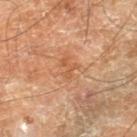This lesion was catalogued during total-body skin photography and was not selected for biopsy.
A roughly 15 mm field-of-view crop from a total-body skin photograph.
A male subject roughly 70 years of age.
The lesion is on the right lower leg.